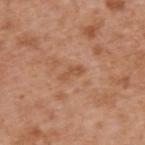Cropped from a whole-body photographic skin survey; the tile spans about 15 mm. A male patient, in their mid-60s. Captured under white-light illumination. The lesion is located on the upper back. Approximately 2.5 mm at its widest.